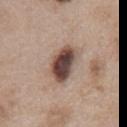Q: Was this lesion biopsied?
A: total-body-photography surveillance lesion; no biopsy
Q: Where on the body is the lesion?
A: the chest
Q: What kind of image is this?
A: total-body-photography crop, ~15 mm field of view
Q: Lesion size?
A: about 5 mm
Q: What did automated image analysis measure?
A: an average lesion color of about L≈46 a*≈17 b*≈23 (CIELAB), a lesion–skin lightness drop of about 19, and a lesion-to-skin contrast of about 13 (normalized; higher = more distinct); a border-irregularity rating of about 2/10, a within-lesion color-variation index near 8.5/10, and radial color variation of about 2.5; an automated nevus-likeness rating near 80 out of 100
Q: Patient demographics?
A: female, aged around 40